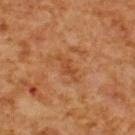Clinical impression: No biopsy was performed on this lesion — it was imaged during a full skin examination and was not determined to be concerning. Acquisition and patient details: A 15 mm crop from a total-body photograph taken for skin-cancer surveillance. Measured at roughly 3.5 mm in maximum diameter. The tile uses cross-polarized illumination. From the upper back. A male subject, aged 58–62.